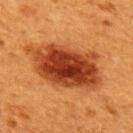The lesion was tiled from a total-body skin photograph and was not biopsied. From the upper back. The recorded lesion diameter is about 9 mm. This is a cross-polarized tile. A female subject in their 40s. A roughly 15 mm field-of-view crop from a total-body skin photograph. Automated tile analysis of the lesion measured a lesion color around L≈35 a*≈27 b*≈35 in CIELAB and a normalized border contrast of about 12. It also reported a classifier nevus-likeness of about 100/100.